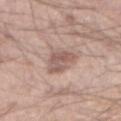| key | value |
|---|---|
| biopsy status | total-body-photography surveillance lesion; no biopsy |
| image source | ~15 mm crop, total-body skin-cancer survey |
| site | the right forearm |
| subject | male, aged around 45 |